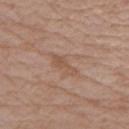Q: Is there a histopathology result?
A: no biopsy performed (imaged during a skin exam)
Q: What is the lesion's diameter?
A: ~3.5 mm (longest diameter)
Q: What are the patient's age and sex?
A: female, in their mid-50s
Q: What is the anatomic site?
A: the arm
Q: What is the imaging modality?
A: total-body-photography crop, ~15 mm field of view